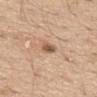Assessment: No biopsy was performed on this lesion — it was imaged during a full skin examination and was not determined to be concerning. Acquisition and patient details: The recorded lesion diameter is about 2.5 mm. Captured under white-light illumination. Cropped from a whole-body photographic skin survey; the tile spans about 15 mm. From the mid back. A male subject, approximately 70 years of age. The lesion-visualizer software estimated a lesion area of about 3 mm² and an outline eccentricity of about 0.85 (0 = round, 1 = elongated). The software also gave a lesion color around L≈56 a*≈20 b*≈31 in CIELAB, roughly 13 lightness units darker than nearby skin, and a normalized lesion–skin contrast near 8.5. And it measured a border-irregularity rating of about 2/10, a within-lesion color-variation index near 1/10, and a peripheral color-asymmetry measure near 0.5.